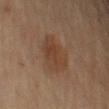This lesion was catalogued during total-body skin photography and was not selected for biopsy. A male subject, about 55 years old. This image is a 15 mm lesion crop taken from a total-body photograph. An algorithmic analysis of the crop reported a footprint of about 14 mm², a shape eccentricity near 0.6, and a shape-asymmetry score of about 0.2 (0 = symmetric). And it measured a mean CIELAB color near L≈37 a*≈16 b*≈27 and a lesion–skin lightness drop of about 6. The analysis additionally found border irregularity of about 2 on a 0–10 scale. The software also gave a detector confidence of about 100 out of 100 that the crop contains a lesion. On the left upper arm.